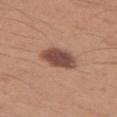biopsy status: no biopsy performed (imaged during a skin exam)
patient: male, in their mid-30s
location: the right upper arm
image: total-body-photography crop, ~15 mm field of view
lesion diameter: about 4 mm
lighting: white-light illumination